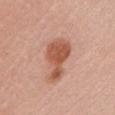Clinical summary: A 15 mm close-up extracted from a 3D total-body photography capture. About 5.5 mm across. The tile uses white-light illumination. A female patient in their 50s. From the head or neck.